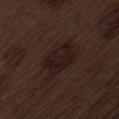follow-up = no biopsy performed (imaged during a skin exam) | tile lighting = white-light | imaging modality = total-body-photography crop, ~15 mm field of view | anatomic site = the lower back | patient = male, aged approximately 70 | automated lesion analysis = a lesion color around L≈17 a*≈13 b*≈15 in CIELAB, a lesion–skin lightness drop of about 5, and a normalized lesion–skin contrast near 8; a nevus-likeness score of about 10/100 and a detector confidence of about 100 out of 100 that the crop contains a lesion.